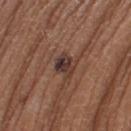Recorded during total-body skin imaging; not selected for excision or biopsy. A 15 mm crop from a total-body photograph taken for skin-cancer surveillance. The patient is a female aged around 35. From the leg.